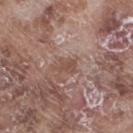Assessment:
This lesion was catalogued during total-body skin photography and was not selected for biopsy.
Context:
A male patient aged 73 to 77. Automated tile analysis of the lesion measured a lesion area of about 3.5 mm² and an outline eccentricity of about 0.75 (0 = round, 1 = elongated). It also reported a border-irregularity index near 3.5/10 and peripheral color asymmetry of about 0.5. And it measured an automated nevus-likeness rating near 0 out of 100 and lesion-presence confidence of about 100/100. The lesion is located on the leg. The recorded lesion diameter is about 2.5 mm. Imaged with white-light lighting. A close-up tile cropped from a whole-body skin photograph, about 15 mm across.